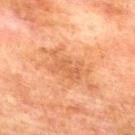biopsy status — catalogued during a skin exam; not biopsied | acquisition — ~15 mm tile from a whole-body skin photo | anatomic site — the upper back | patient — male, aged around 75 | lesion diameter — ~5 mm (longest diameter).